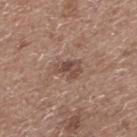Q: Was this lesion biopsied?
A: no biopsy performed (imaged during a skin exam)
Q: Lesion location?
A: the upper back
Q: What did automated image analysis measure?
A: a lesion area of about 5.5 mm², an outline eccentricity of about 0.75 (0 = round, 1 = elongated), and two-axis asymmetry of about 0.35; a classifier nevus-likeness of about 5/100 and lesion-presence confidence of about 100/100
Q: What are the patient's age and sex?
A: male, aged approximately 60
Q: How was this image acquired?
A: ~15 mm crop, total-body skin-cancer survey